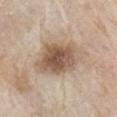Clinical summary:
Located on the right upper arm. This is a white-light tile. Cropped from a whole-body photographic skin survey; the tile spans about 15 mm. A male patient, about 65 years old.
Conclusion:
Histopathologically confirmed as a melanoma in situ.